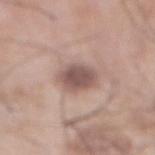Part of a total-body skin-imaging series; this lesion was reviewed on a skin check and was not flagged for biopsy. A male patient aged 68 to 72. From the abdomen. The lesion's longest dimension is about 4 mm. The tile uses white-light illumination. A lesion tile, about 15 mm wide, cut from a 3D total-body photograph.